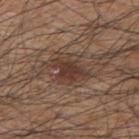  biopsy_status: not biopsied; imaged during a skin examination
  lighting: white-light
  lesion_size:
    long_diameter_mm_approx: 4.0
  site: upper back
  patient:
    sex: male
    age_approx: 45
  image:
    source: total-body photography crop
    field_of_view_mm: 15